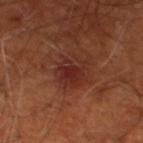{
  "biopsy_status": "not biopsied; imaged during a skin examination",
  "site": "left lower leg",
  "lighting": "cross-polarized",
  "patient": {
    "age_approx": 65
  },
  "image": {
    "source": "total-body photography crop",
    "field_of_view_mm": 15
  }
}